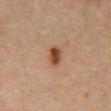Part of a total-body skin-imaging series; this lesion was reviewed on a skin check and was not flagged for biopsy. The lesion is located on the abdomen. The total-body-photography lesion software estimated a peripheral color-asymmetry measure near 2. The software also gave an automated nevus-likeness rating near 100 out of 100. A male subject aged 63–67. This image is a 15 mm lesion crop taken from a total-body photograph.